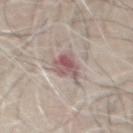Imaged during a routine full-body skin examination; the lesion was not biopsied and no histopathology is available. A male subject, about 65 years old. Located on the chest. A roughly 15 mm field-of-view crop from a total-body skin photograph. The total-body-photography lesion software estimated a lesion color around L≈56 a*≈18 b*≈18 in CIELAB and roughly 12 lightness units darker than nearby skin. It also reported a nevus-likeness score of about 0/100 and a detector confidence of about 55 out of 100 that the crop contains a lesion. The recorded lesion diameter is about 3.5 mm.